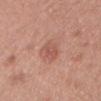Recorded during total-body skin imaging; not selected for excision or biopsy. Automated image analysis of the tile measured border irregularity of about 2.5 on a 0–10 scale and a within-lesion color-variation index near 2/10. The recorded lesion diameter is about 3 mm. This image is a 15 mm lesion crop taken from a total-body photograph. A female patient, approximately 40 years of age. Located on the chest.